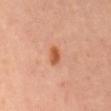Part of a total-body skin-imaging series; this lesion was reviewed on a skin check and was not flagged for biopsy. A female subject about 65 years old. A 15 mm close-up tile from a total-body photography series done for melanoma screening. On the mid back.